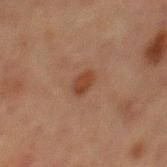Impression: The lesion was tiled from a total-body skin photograph and was not biopsied. Context: This is a cross-polarized tile. The total-body-photography lesion software estimated a lesion color around L≈33 a*≈19 b*≈26 in CIELAB, a lesion–skin lightness drop of about 7, and a normalized border contrast of about 7.5. It also reported an automated nevus-likeness rating near 90 out of 100 and lesion-presence confidence of about 100/100. Cropped from a whole-body photographic skin survey; the tile spans about 15 mm. About 2.5 mm across. On the abdomen. A male subject, about 65 years old.